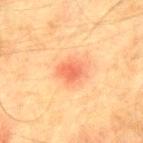{"biopsy_status": "not biopsied; imaged during a skin examination"}